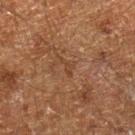Findings:
* biopsy status — total-body-photography surveillance lesion; no biopsy